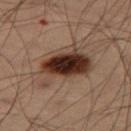biopsy_status: not biopsied; imaged during a skin examination
automated_metrics:
  cielab_L: 27
  cielab_a: 15
  cielab_b: 20
  vs_skin_darker_L: 13.0
  vs_skin_contrast_norm: 13.5
  border_irregularity_0_10: 4.0
  color_variation_0_10: 8.5
  nevus_likeness_0_100: 100
  lesion_detection_confidence_0_100: 100
site: right thigh
image:
  source: total-body photography crop
  field_of_view_mm: 15
lighting: cross-polarized
patient:
  sex: male
  age_approx: 55
lesion_size:
  long_diameter_mm_approx: 6.5